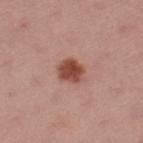Impression:
Captured during whole-body skin photography for melanoma surveillance; the lesion was not biopsied.
Background:
The patient is a female in their mid- to late 50s. Imaged with white-light lighting. This image is a 15 mm lesion crop taken from a total-body photograph. About 3 mm across. The lesion is located on the left thigh. The lesion-visualizer software estimated an outline eccentricity of about 0.55 (0 = round, 1 = elongated) and a symmetry-axis asymmetry near 0.15. It also reported a classifier nevus-likeness of about 100/100 and lesion-presence confidence of about 100/100.